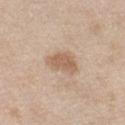| feature | finding |
|---|---|
| workup | catalogued during a skin exam; not biopsied |